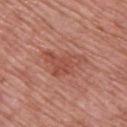Assessment:
The lesion was tiled from a total-body skin photograph and was not biopsied.
Background:
From the upper back. The lesion-visualizer software estimated a footprint of about 10 mm² and a shape-asymmetry score of about 0.55 (0 = symmetric). The software also gave a mean CIELAB color near L≈50 a*≈28 b*≈29, about 8 CIELAB-L* units darker than the surrounding skin, and a normalized lesion–skin contrast near 6. The subject is a male roughly 50 years of age. Imaged with white-light lighting. About 6 mm across. A roughly 15 mm field-of-view crop from a total-body skin photograph.